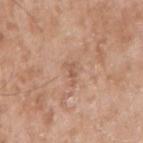| key | value |
|---|---|
| notes | total-body-photography surveillance lesion; no biopsy |
| location | the arm |
| lighting | white-light |
| patient | male, aged around 50 |
| imaging modality | ~15 mm crop, total-body skin-cancer survey |
| diameter | ~2.5 mm (longest diameter) |